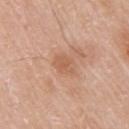Assessment: The lesion was tiled from a total-body skin photograph and was not biopsied. Acquisition and patient details: The recorded lesion diameter is about 3 mm. Captured under white-light illumination. Located on the right upper arm. An algorithmic analysis of the crop reported an eccentricity of roughly 0.65. It also reported about 7 CIELAB-L* units darker than the surrounding skin and a lesion-to-skin contrast of about 5 (normalized; higher = more distinct). It also reported a nevus-likeness score of about 0/100. A 15 mm close-up extracted from a 3D total-body photography capture. A female subject in their mid-50s.